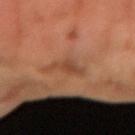Recorded during total-body skin imaging; not selected for excision or biopsy. A 15 mm close-up tile from a total-body photography series done for melanoma screening. Approximately 3.5 mm at its widest. The tile uses cross-polarized illumination. The patient is a male approximately 65 years of age. The total-body-photography lesion software estimated an automated nevus-likeness rating near 0 out of 100 and a lesion-detection confidence of about 100/100.